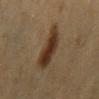<record>
  <site>abdomen</site>
  <lesion_size>
    <long_diameter_mm_approx>5.5</long_diameter_mm_approx>
  </lesion_size>
  <patient>
    <sex>female</sex>
    <age_approx>40</age_approx>
  </patient>
  <automated_metrics>
    <nevus_likeness_0_100>95</nevus_likeness_0_100>
    <lesion_detection_confidence_0_100>100</lesion_detection_confidence_0_100>
  </automated_metrics>
  <lighting>cross-polarized</lighting>
  <image>
    <source>total-body photography crop</source>
    <field_of_view_mm>15</field_of_view_mm>
  </image>
</record>